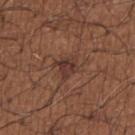Impression:
Recorded during total-body skin imaging; not selected for excision or biopsy.
Acquisition and patient details:
A 15 mm close-up tile from a total-body photography series done for melanoma screening. The patient is a male aged around 65. From the right upper arm.